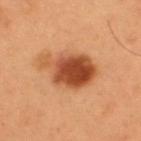Automated tile analysis of the lesion measured a mean CIELAB color near L≈48 a*≈28 b*≈38, about 16 CIELAB-L* units darker than the surrounding skin, and a normalized border contrast of about 11. And it measured a color-variation rating of about 5.5/10 and radial color variation of about 1.5. On the upper back. Approximately 6.5 mm at its widest. Imaged with cross-polarized lighting. A male subject aged 53–57. A region of skin cropped from a whole-body photographic capture, roughly 15 mm wide.